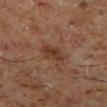Part of a total-body skin-imaging series; this lesion was reviewed on a skin check and was not flagged for biopsy. A region of skin cropped from a whole-body photographic capture, roughly 15 mm wide. Longest diameter approximately 3 mm. From the left lower leg. The patient is a male in their 60s. Captured under cross-polarized illumination.